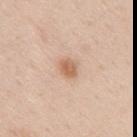No biopsy was performed on this lesion — it was imaged during a full skin examination and was not determined to be concerning.
A female patient, aged 28–32.
A roughly 15 mm field-of-view crop from a total-body skin photograph.
On the upper back.